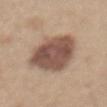Part of a total-body skin-imaging series; this lesion was reviewed on a skin check and was not flagged for biopsy. Imaged with white-light lighting. A close-up tile cropped from a whole-body skin photograph, about 15 mm across. About 8.5 mm across. A male patient, in their 30s. The lesion is on the chest.